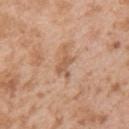Notes:
• workup — imaged on a skin check; not biopsied
• subject — male, about 25 years old
• size — about 3 mm
• lighting — white-light
• anatomic site — the upper back
• image source — 15 mm crop, total-body photography